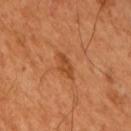Q: Is there a histopathology result?
A: imaged on a skin check; not biopsied
Q: Patient demographics?
A: male, in their 50s
Q: What is the lesion's diameter?
A: ≈2.5 mm
Q: What is the anatomic site?
A: the right upper arm
Q: Automated lesion metrics?
A: an outline eccentricity of about 0.8 (0 = round, 1 = elongated) and two-axis asymmetry of about 0.25; a classifier nevus-likeness of about 15/100
Q: What is the imaging modality?
A: ~15 mm crop, total-body skin-cancer survey
Q: How was the tile lit?
A: cross-polarized illumination